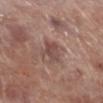Background:
A male subject, aged approximately 70. From the right lower leg. About 3.5 mm across. This image is a 15 mm lesion crop taken from a total-body photograph. Imaged with white-light lighting.